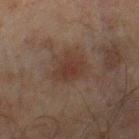The lesion was tiled from a total-body skin photograph and was not biopsied. Longest diameter approximately 3.5 mm. A male subject roughly 45 years of age. Cropped from a total-body skin-imaging series; the visible field is about 15 mm. On the right lower leg. The tile uses cross-polarized illumination. The lesion-visualizer software estimated a within-lesion color-variation index near 2/10 and radial color variation of about 0.5.